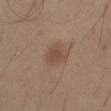Q: Is there a histopathology result?
A: imaged on a skin check; not biopsied
Q: What is the anatomic site?
A: the right thigh
Q: What kind of image is this?
A: 15 mm crop, total-body photography
Q: Who is the patient?
A: male, aged approximately 55
Q: Automated lesion metrics?
A: a shape eccentricity near 0.65 and a shape-asymmetry score of about 0.4 (0 = symmetric); a border-irregularity index near 3.5/10, internal color variation of about 1.5 on a 0–10 scale, and radial color variation of about 0.5; an automated nevus-likeness rating near 85 out of 100 and a lesion-detection confidence of about 100/100
Q: Lesion size?
A: ≈3 mm
Q: Illumination type?
A: cross-polarized illumination